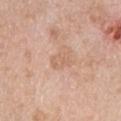The lesion-visualizer software estimated an automated nevus-likeness rating near 0 out of 100. A female patient aged 68–72. Captured under white-light illumination. A 15 mm close-up extracted from a 3D total-body photography capture. Longest diameter approximately 2.5 mm. On the left lower leg.